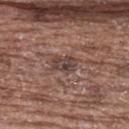Q: Automated lesion metrics?
A: a shape eccentricity near 0.75 and two-axis asymmetry of about 0.2
Q: Where on the body is the lesion?
A: the upper back
Q: Who is the patient?
A: female, about 65 years old
Q: How large is the lesion?
A: ≈3 mm
Q: Illumination type?
A: white-light illumination
Q: How was this image acquired?
A: ~15 mm crop, total-body skin-cancer survey